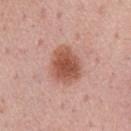{"biopsy_status": "not biopsied; imaged during a skin examination", "lesion_size": {"long_diameter_mm_approx": 4.5}, "site": "chest", "patient": {"sex": "male", "age_approx": 75}, "lighting": "white-light", "image": {"source": "total-body photography crop", "field_of_view_mm": 15}}